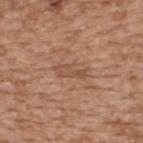Part of a total-body skin-imaging series; this lesion was reviewed on a skin check and was not flagged for biopsy.
This is a white-light tile.
A 15 mm close-up tile from a total-body photography series done for melanoma screening.
Located on the upper back.
Approximately 4 mm at its widest.
A male patient aged 63–67.
An algorithmic analysis of the crop reported an area of roughly 5 mm², a shape eccentricity near 0.9, and a shape-asymmetry score of about 0.4 (0 = symmetric). It also reported an average lesion color of about L≈51 a*≈21 b*≈31 (CIELAB) and about 7 CIELAB-L* units darker than the surrounding skin.